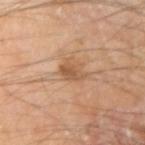{
  "biopsy_status": "not biopsied; imaged during a skin examination",
  "lighting": "cross-polarized",
  "image": {
    "source": "total-body photography crop",
    "field_of_view_mm": 15
  },
  "patient": {
    "sex": "male",
    "age_approx": 65
  },
  "site": "right upper arm"
}